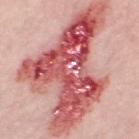The lesion was tiled from a total-body skin photograph and was not biopsied. From the leg. A female patient, aged 48 to 52. The lesion's longest dimension is about 14.5 mm. A 15 mm close-up tile from a total-body photography series done for melanoma screening.